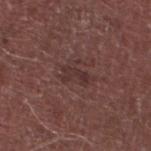notes — catalogued during a skin exam; not biopsied | image — ~15 mm tile from a whole-body skin photo | illumination — white-light illumination | patient — male, roughly 75 years of age | size — ≈3 mm | image-analysis metrics — a mean CIELAB color near L≈32 a*≈19 b*≈18, roughly 6 lightness units darker than nearby skin, and a lesion-to-skin contrast of about 6.5 (normalized; higher = more distinct); a border-irregularity index near 5.5/10 and a peripheral color-asymmetry measure near 0; a classifier nevus-likeness of about 0/100 and a lesion-detection confidence of about 100/100 | anatomic site — the left lower leg.